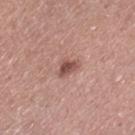workup = imaged on a skin check; not biopsied | lesion size = about 2.5 mm | image = 15 mm crop, total-body photography | lighting = white-light | patient = female, aged 48 to 52 | site = the left thigh.